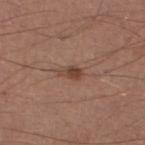Imaged during a routine full-body skin examination; the lesion was not biopsied and no histopathology is available. A male subject aged 53–57. A 15 mm close-up tile from a total-body photography series done for melanoma screening. The lesion is on the left lower leg. Imaged with white-light lighting. The total-body-photography lesion software estimated a lesion area of about 3 mm², a shape eccentricity near 0.8, and a shape-asymmetry score of about 0.35 (0 = symmetric). The software also gave border irregularity of about 3 on a 0–10 scale. Longest diameter approximately 2.5 mm.